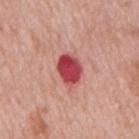Clinical impression:
The lesion was tiled from a total-body skin photograph and was not biopsied.
Background:
Captured under white-light illumination. The subject is a male about 60 years old. A lesion tile, about 15 mm wide, cut from a 3D total-body photograph. Located on the mid back.